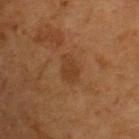Impression:
No biopsy was performed on this lesion — it was imaged during a full skin examination and was not determined to be concerning.
Context:
Measured at roughly 3 mm in maximum diameter. On the upper back. The tile uses cross-polarized illumination. The subject is a female in their 40s. A 15 mm close-up extracted from a 3D total-body photography capture.